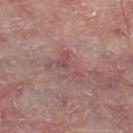Findings:
• workup: no biopsy performed (imaged during a skin exam)
• patient: male, aged approximately 60
• illumination: cross-polarized illumination
• image source: total-body-photography crop, ~15 mm field of view
• image-analysis metrics: a footprint of about 7 mm² and an outline eccentricity of about 0.85 (0 = round, 1 = elongated); a border-irregularity rating of about 8/10, a within-lesion color-variation index near 4/10, and radial color variation of about 1.5; a classifier nevus-likeness of about 0/100
• diameter: about 4.5 mm
• anatomic site: the right lower leg